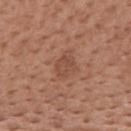{
  "image": {
    "source": "total-body photography crop",
    "field_of_view_mm": 15
  },
  "automated_metrics": {
    "area_mm2_approx": 6.0,
    "eccentricity": 0.7,
    "cielab_L": 48,
    "cielab_a": 23,
    "cielab_b": 29,
    "vs_skin_darker_L": 7.0,
    "vs_skin_contrast_norm": 5.5,
    "color_variation_0_10": 2.0,
    "peripheral_color_asymmetry": 0.5,
    "nevus_likeness_0_100": 0
  },
  "lesion_size": {
    "long_diameter_mm_approx": 3.0
  },
  "lighting": "white-light",
  "patient": {
    "sex": "male",
    "age_approx": 55
  },
  "site": "mid back"
}